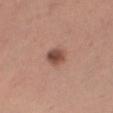workup: no biopsy performed (imaged during a skin exam).